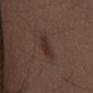Case summary:
- workup: catalogued during a skin exam; not biopsied
- patient: male, in their 50s
- lesion diameter: about 4 mm
- image source: total-body-photography crop, ~15 mm field of view
- body site: the front of the torso